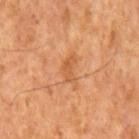This lesion was catalogued during total-body skin photography and was not selected for biopsy.